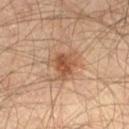<case>
<biopsy_status>not biopsied; imaged during a skin examination</biopsy_status>
<patient>
  <sex>male</sex>
  <age_approx>65</age_approx>
</patient>
<lesion_size>
  <long_diameter_mm_approx>4.0</long_diameter_mm_approx>
</lesion_size>
<image>
  <source>total-body photography crop</source>
  <field_of_view_mm>15</field_of_view_mm>
</image>
<site>right thigh</site>
<lighting>cross-polarized</lighting>
</case>